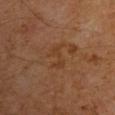This lesion was catalogued during total-body skin photography and was not selected for biopsy. A male subject approximately 60 years of age. On the right upper arm. The tile uses cross-polarized illumination. A 15 mm close-up tile from a total-body photography series done for melanoma screening.